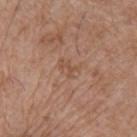<case>
<biopsy_status>not biopsied; imaged during a skin examination</biopsy_status>
<image>
  <source>total-body photography crop</source>
  <field_of_view_mm>15</field_of_view_mm>
</image>
<lesion_size>
  <long_diameter_mm_approx>2.5</long_diameter_mm_approx>
</lesion_size>
<site>chest</site>
<patient>
  <sex>male</sex>
  <age_approx>65</age_approx>
</patient>
</case>